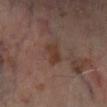Q: Is there a histopathology result?
A: no biopsy performed (imaged during a skin exam)
Q: What kind of image is this?
A: ~15 mm crop, total-body skin-cancer survey
Q: What is the lesion's diameter?
A: about 3 mm
Q: What are the patient's age and sex?
A: male, aged 68–72
Q: Lesion location?
A: the right lower leg